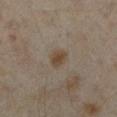{"biopsy_status": "not biopsied; imaged during a skin examination", "patient": {"sex": "female", "age_approx": 35}, "site": "left lower leg", "image": {"source": "total-body photography crop", "field_of_view_mm": 15}}